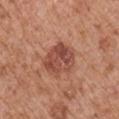This lesion was catalogued during total-body skin photography and was not selected for biopsy.
The tile uses white-light illumination.
The lesion's longest dimension is about 4.5 mm.
A male patient aged around 65.
A 15 mm crop from a total-body photograph taken for skin-cancer surveillance.
An algorithmic analysis of the crop reported a shape eccentricity near 0.55 and a shape-asymmetry score of about 0.15 (0 = symmetric). The software also gave a border-irregularity rating of about 2/10, a color-variation rating of about 5.5/10, and radial color variation of about 2.
The lesion is located on the left upper arm.